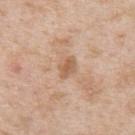  biopsy_status: not biopsied; imaged during a skin examination
  image:
    source: total-body photography crop
    field_of_view_mm: 15
  automated_metrics:
    area_mm2_approx: 3.5
    shape_asymmetry: 0.25
    border_irregularity_0_10: 2.0
    color_variation_0_10: 1.5
    peripheral_color_asymmetry: 0.5
  lighting: white-light
  site: right upper arm
  lesion_size:
    long_diameter_mm_approx: 2.5
  patient:
    sex: male
    age_approx: 55